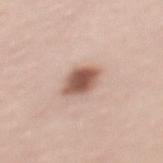notes: total-body-photography surveillance lesion; no biopsy | illumination: white-light illumination | size: about 3.5 mm | anatomic site: the mid back | image source: 15 mm crop, total-body photography | patient: female, aged approximately 40.